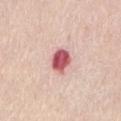This lesion was catalogued during total-body skin photography and was not selected for biopsy. From the abdomen. The patient is a female roughly 65 years of age. This image is a 15 mm lesion crop taken from a total-body photograph. Longest diameter approximately 3 mm. Imaged with white-light lighting.